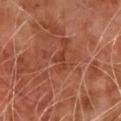<case>
  <biopsy_status>not biopsied; imaged during a skin examination</biopsy_status>
  <patient>
    <sex>male</sex>
    <age_approx>65</age_approx>
  </patient>
  <image>
    <source>total-body photography crop</source>
    <field_of_view_mm>15</field_of_view_mm>
  </image>
</case>